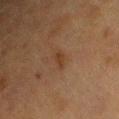Imaged during a routine full-body skin examination; the lesion was not biopsied and no histopathology is available. The subject is a female approximately 55 years of age. The total-body-photography lesion software estimated a footprint of about 3 mm², a shape eccentricity near 0.9, and two-axis asymmetry of about 0.25. The analysis additionally found a mean CIELAB color near L≈31 a*≈16 b*≈27, a lesion–skin lightness drop of about 5, and a normalized border contrast of about 6. Approximately 3 mm at its widest. Imaged with cross-polarized lighting. A 15 mm crop from a total-body photograph taken for skin-cancer surveillance. Located on the right upper arm.